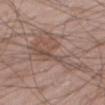{
  "biopsy_status": "not biopsied; imaged during a skin examination",
  "site": "leg",
  "image": {
    "source": "total-body photography crop",
    "field_of_view_mm": 15
  },
  "automated_metrics": {
    "cielab_L": 50,
    "cielab_a": 17,
    "cielab_b": 24,
    "vs_skin_darker_L": 8.0,
    "vs_skin_contrast_norm": 6.0,
    "color_variation_0_10": 5.0,
    "peripheral_color_asymmetry": 2.0,
    "nevus_likeness_0_100": 0
  },
  "patient": {
    "sex": "male",
    "age_approx": 60
  }
}